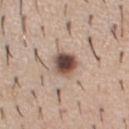biopsy status: imaged on a skin check; not biopsied | subject: male, about 40 years old | lighting: white-light | acquisition: 15 mm crop, total-body photography | anatomic site: the chest.